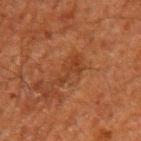Part of a total-body skin-imaging series; this lesion was reviewed on a skin check and was not flagged for biopsy.